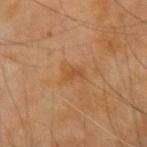The lesion was tiled from a total-body skin photograph and was not biopsied.
The patient is a male about 70 years old.
A 15 mm crop from a total-body photograph taken for skin-cancer surveillance.
The lesion is located on the right forearm.
The lesion's longest dimension is about 2.5 mm.